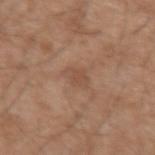The lesion was photographed on a routine skin check and not biopsied; there is no pathology result. A roughly 15 mm field-of-view crop from a total-body skin photograph. A male patient, aged 48–52. The lesion is on the arm. Captured under white-light illumination.